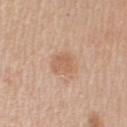Imaged during a routine full-body skin examination; the lesion was not biopsied and no histopathology is available. Cropped from a whole-body photographic skin survey; the tile spans about 15 mm. A male patient aged 58 to 62. Automated image analysis of the tile measured an eccentricity of roughly 0.6 and two-axis asymmetry of about 0.3. And it measured about 8 CIELAB-L* units darker than the surrounding skin and a normalized border contrast of about 5.5. The analysis additionally found a border-irregularity index near 3/10. The lesion is on the left upper arm.